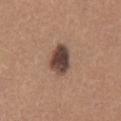| feature | finding |
|---|---|
| workup | no biopsy performed (imaged during a skin exam) |
| lighting | white-light |
| patient | male, in their 30s |
| automated metrics | an area of roughly 8.5 mm², an eccentricity of roughly 0.8, and a symmetry-axis asymmetry near 0.2; a nevus-likeness score of about 70/100 and lesion-presence confidence of about 100/100 |
| imaging modality | ~15 mm crop, total-body skin-cancer survey |
| anatomic site | the back |
| lesion size | ~4.5 mm (longest diameter) |